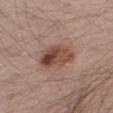biopsy status: imaged on a skin check; not biopsied | subject: male, aged 38 to 42 | image: 15 mm crop, total-body photography | anatomic site: the leg.